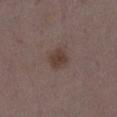Clinical impression: Part of a total-body skin-imaging series; this lesion was reviewed on a skin check and was not flagged for biopsy. Background: A female subject, aged approximately 35. Measured at roughly 2.5 mm in maximum diameter. This image is a 15 mm lesion crop taken from a total-body photograph. Located on the abdomen. The lesion-visualizer software estimated a lesion area of about 5 mm², a shape eccentricity near 0.5, and two-axis asymmetry of about 0.2. And it measured a lesion color around L≈38 a*≈15 b*≈22 in CIELAB, about 8 CIELAB-L* units darker than the surrounding skin, and a normalized lesion–skin contrast near 8. The analysis additionally found lesion-presence confidence of about 100/100. Imaged with white-light lighting.